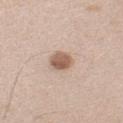<record>
  <biopsy_status>not biopsied; imaged during a skin examination</biopsy_status>
  <lighting>white-light</lighting>
  <image>
    <source>total-body photography crop</source>
    <field_of_view_mm>15</field_of_view_mm>
  </image>
  <site>left upper arm</site>
  <patient>
    <sex>male</sex>
    <age_approx>50</age_approx>
  </patient>
</record>